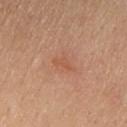The lesion was photographed on a routine skin check and not biopsied; there is no pathology result. A 15 mm crop from a total-body photograph taken for skin-cancer surveillance. The lesion is located on the upper back. A female subject aged approximately 45.